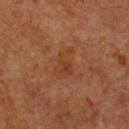The lesion was photographed on a routine skin check and not biopsied; there is no pathology result. Captured under cross-polarized illumination. On the chest. A roughly 15 mm field-of-view crop from a total-body skin photograph. About 4 mm across. A male patient, aged around 75.